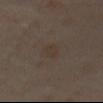Notes:
– workup · no biopsy performed (imaged during a skin exam)
– acquisition · ~15 mm tile from a whole-body skin photo
– size · ~3.5 mm (longest diameter)
– location · the chest
– subject · male, in their mid-60s
– lighting · cross-polarized